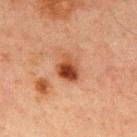| field | value |
|---|---|
| notes | imaged on a skin check; not biopsied |
| image | total-body-photography crop, ~15 mm field of view |
| body site | the chest |
| subject | male, aged approximately 65 |